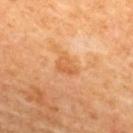Part of a total-body skin-imaging series; this lesion was reviewed on a skin check and was not flagged for biopsy.
A lesion tile, about 15 mm wide, cut from a 3D total-body photograph.
Approximately 3 mm at its widest.
From the back.
The subject is a female in their mid-60s.